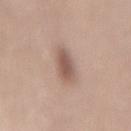Part of a total-body skin-imaging series; this lesion was reviewed on a skin check and was not flagged for biopsy. On the mid back. The lesion-visualizer software estimated a footprint of about 7 mm², a shape eccentricity near 0.75, and two-axis asymmetry of about 0.2. The analysis additionally found peripheral color asymmetry of about 1. The patient is a male aged around 70. A 15 mm crop from a total-body photograph taken for skin-cancer surveillance. The tile uses white-light illumination.